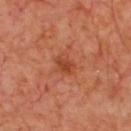follow-up: catalogued during a skin exam; not biopsied
body site: the front of the torso
subject: male, in their mid- to late 60s
imaging modality: ~15 mm tile from a whole-body skin photo